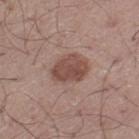{"biopsy_status": "not biopsied; imaged during a skin examination", "patient": {"sex": "male", "age_approx": 50}, "automated_metrics": {"area_mm2_approx": 11.0, "eccentricity": 0.7, "shape_asymmetry": 0.2, "cielab_L": 48, "cielab_a": 20, "cielab_b": 24, "vs_skin_darker_L": 11.0, "nevus_likeness_0_100": 30, "lesion_detection_confidence_0_100": 100}, "site": "left thigh", "image": {"source": "total-body photography crop", "field_of_view_mm": 15}}